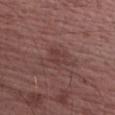The lesion was tiled from a total-body skin photograph and was not biopsied. A male subject, aged 63–67. A 15 mm crop from a total-body photograph taken for skin-cancer surveillance. The lesion's longest dimension is about 2.5 mm. Automated tile analysis of the lesion measured a lesion area of about 4.5 mm², an outline eccentricity of about 0.6 (0 = round, 1 = elongated), and a shape-asymmetry score of about 0.3 (0 = symmetric). The analysis additionally found a nevus-likeness score of about 0/100 and a lesion-detection confidence of about 100/100. The lesion is on the right upper arm. Captured under white-light illumination.